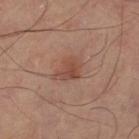Q: How large is the lesion?
A: ~3.5 mm (longest diameter)
Q: What lighting was used for the tile?
A: cross-polarized
Q: What did automated image analysis measure?
A: an area of roughly 6.5 mm² and a shape-asymmetry score of about 0.35 (0 = symmetric); an average lesion color of about L≈48 a*≈22 b*≈28 (CIELAB), about 8 CIELAB-L* units darker than the surrounding skin, and a normalized lesion–skin contrast near 6.5; border irregularity of about 3.5 on a 0–10 scale, internal color variation of about 4 on a 0–10 scale, and peripheral color asymmetry of about 1.5; a classifier nevus-likeness of about 70/100 and a lesion-detection confidence of about 100/100
Q: What is the anatomic site?
A: the left thigh
Q: What kind of image is this?
A: 15 mm crop, total-body photography